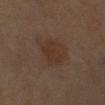Clinical impression:
Recorded during total-body skin imaging; not selected for excision or biopsy.
Context:
Automated tile analysis of the lesion measured an eccentricity of roughly 0.75 and a symmetry-axis asymmetry near 0.3. The software also gave a lesion color around L≈31 a*≈15 b*≈24 in CIELAB, about 5 CIELAB-L* units darker than the surrounding skin, and a normalized lesion–skin contrast near 6. Located on the left forearm. A 15 mm crop from a total-body photograph taken for skin-cancer surveillance. A male subject aged around 70. This is a cross-polarized tile.